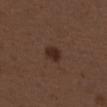Q: What did automated image analysis measure?
A: a lesion area of about 3.5 mm² and an eccentricity of roughly 0.75; border irregularity of about 1.5 on a 0–10 scale, a color-variation rating of about 1.5/10, and a peripheral color-asymmetry measure near 0.5; a classifier nevus-likeness of about 85/100 and lesion-presence confidence of about 100/100
Q: What lighting was used for the tile?
A: white-light
Q: Who is the patient?
A: female, about 50 years old
Q: Lesion location?
A: the back
Q: How was this image acquired?
A: 15 mm crop, total-body photography